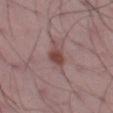  biopsy_status: not biopsied; imaged during a skin examination
  lesion_size:
    long_diameter_mm_approx: 3.5
  automated_metrics:
    area_mm2_approx: 5.0
    eccentricity: 0.75
    border_irregularity_0_10: 2.5
    color_variation_0_10: 2.0
    nevus_likeness_0_100: 85
  image:
    source: total-body photography crop
    field_of_view_mm: 15
  lighting: white-light
  site: left thigh
  patient:
    sex: male
    age_approx: 50